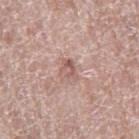Captured during whole-body skin photography for melanoma surveillance; the lesion was not biopsied. A female patient, aged approximately 60. Cropped from a whole-body photographic skin survey; the tile spans about 15 mm. The lesion-visualizer software estimated internal color variation of about 2 on a 0–10 scale and radial color variation of about 1. About 2.5 mm across. On the right thigh. Captured under white-light illumination.